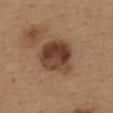This lesion was catalogued during total-body skin photography and was not selected for biopsy. A 15 mm close-up tile from a total-body photography series done for melanoma screening. This is a white-light tile. From the upper back. Longest diameter approximately 5 mm. Automated tile analysis of the lesion measured an area of roughly 17 mm², an eccentricity of roughly 0.4, and a symmetry-axis asymmetry near 0.25. It also reported a classifier nevus-likeness of about 50/100 and lesion-presence confidence of about 100/100. A female patient roughly 40 years of age.